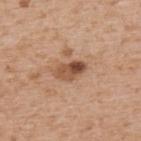Imaged during a routine full-body skin examination; the lesion was not biopsied and no histopathology is available. A male patient, aged 63–67. The lesion is on the back. The lesion's longest dimension is about 3.5 mm. The tile uses white-light illumination. A lesion tile, about 15 mm wide, cut from a 3D total-body photograph.